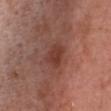<lesion>
<biopsy_status>not biopsied; imaged during a skin examination</biopsy_status>
<patient>
  <sex>female</sex>
  <age_approx>65</age_approx>
</patient>
<automated_metrics>
  <area_mm2_approx>6.0</area_mm2_approx>
  <eccentricity>0.65</eccentricity>
  <cielab_L>39</cielab_L>
  <cielab_a>24</cielab_a>
  <cielab_b>27</cielab_b>
  <vs_skin_darker_L>8.0</vs_skin_darker_L>
  <vs_skin_contrast_norm>7.0</vs_skin_contrast_norm>
  <nevus_likeness_0_100>30</nevus_likeness_0_100>
  <lesion_detection_confidence_0_100>100</lesion_detection_confidence_0_100>
</automated_metrics>
<site>front of the torso</site>
<image>
  <source>total-body photography crop</source>
  <field_of_view_mm>15</field_of_view_mm>
</image>
<lighting>white-light</lighting>
<lesion_size>
  <long_diameter_mm_approx>3.0</long_diameter_mm_approx>
</lesion_size>
</lesion>